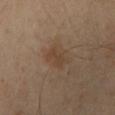{
  "patient": {
    "sex": "male",
    "age_approx": 60
  },
  "lesion_size": {
    "long_diameter_mm_approx": 3.0
  },
  "image": {
    "source": "total-body photography crop",
    "field_of_view_mm": 15
  },
  "site": "chest",
  "lighting": "cross-polarized"
}